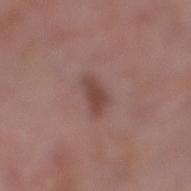biopsy_status: not biopsied; imaged during a skin examination
lighting: white-light
patient:
  sex: female
  age_approx: 70
site: right lower leg
image:
  source: total-body photography crop
  field_of_view_mm: 15
lesion_size:
  long_diameter_mm_approx: 3.0